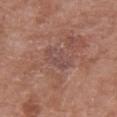Image and clinical context: A female subject, aged 68 to 72. On the chest. A close-up tile cropped from a whole-body skin photograph, about 15 mm across.